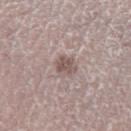Clinical impression: Imaged during a routine full-body skin examination; the lesion was not biopsied and no histopathology is available. Image and clinical context: The tile uses white-light illumination. Measured at roughly 2.5 mm in maximum diameter. The patient is a female roughly 50 years of age. An algorithmic analysis of the crop reported a border-irregularity index near 3/10, a color-variation rating of about 2/10, and peripheral color asymmetry of about 1. A 15 mm crop from a total-body photograph taken for skin-cancer surveillance. The lesion is on the left lower leg.